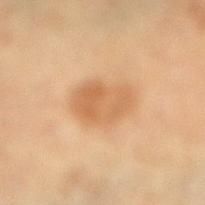Q: Was a biopsy performed?
A: imaged on a skin check; not biopsied
Q: How large is the lesion?
A: about 5 mm
Q: Who is the patient?
A: female, in their 60s
Q: What is the anatomic site?
A: the right lower leg
Q: What kind of image is this?
A: 15 mm crop, total-body photography